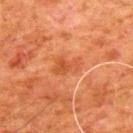This lesion was catalogued during total-body skin photography and was not selected for biopsy.
The lesion is located on the upper back.
A roughly 15 mm field-of-view crop from a total-body skin photograph.
Imaged with cross-polarized lighting.
The lesion's longest dimension is about 3.5 mm.
The patient is a male aged around 80.